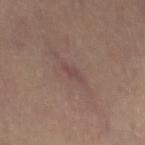notes=no biopsy performed (imaged during a skin exam) | acquisition=~15 mm tile from a whole-body skin photo | tile lighting=cross-polarized illumination | diameter=≈2.5 mm | site=the leg | patient=female, aged 63 to 67.